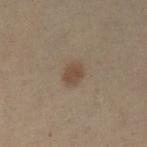Impression: The lesion was photographed on a routine skin check and not biopsied; there is no pathology result. Background: Cropped from a total-body skin-imaging series; the visible field is about 15 mm. The lesion is located on the leg. About 3 mm across. A female subject, aged around 50.